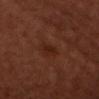Q: Was a biopsy performed?
A: imaged on a skin check; not biopsied
Q: What is the lesion's diameter?
A: ≈2.5 mm
Q: What kind of image is this?
A: total-body-photography crop, ~15 mm field of view
Q: What are the patient's age and sex?
A: female, aged around 50